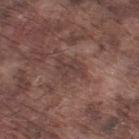| key | value |
|---|---|
| biopsy status | total-body-photography surveillance lesion; no biopsy |
| image | 15 mm crop, total-body photography |
| subject | male, in their mid-70s |
| tile lighting | white-light illumination |
| location | the left thigh |